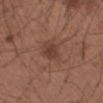Part of a total-body skin-imaging series; this lesion was reviewed on a skin check and was not flagged for biopsy. A male subject, aged 53 to 57. The lesion is located on the left forearm. A region of skin cropped from a whole-body photographic capture, roughly 15 mm wide.